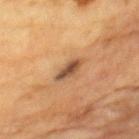A male subject, approximately 85 years of age or older.
Automated tile analysis of the lesion measured a footprint of about 5 mm² and a shape-asymmetry score of about 0.2 (0 = symmetric). The software also gave a normalized border contrast of about 9.5. It also reported a border-irregularity index near 2.5/10, internal color variation of about 2.5 on a 0–10 scale, and a peripheral color-asymmetry measure near 1.
A roughly 15 mm field-of-view crop from a total-body skin photograph.
Captured under cross-polarized illumination.
About 3.5 mm across.
Located on the chest.